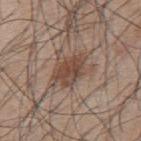Imaged during a routine full-body skin examination; the lesion was not biopsied and no histopathology is available. On the upper back. This is a white-light tile. A 15 mm close-up extracted from a 3D total-body photography capture. A male patient, roughly 55 years of age. The recorded lesion diameter is about 5.5 mm.